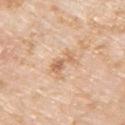<case>
<biopsy_status>not biopsied; imaged during a skin examination</biopsy_status>
<lesion_size>
  <long_diameter_mm_approx>3.0</long_diameter_mm_approx>
</lesion_size>
<automated_metrics>
  <area_mm2_approx>4.5</area_mm2_approx>
  <eccentricity>0.8</eccentricity>
  <shape_asymmetry>0.45</shape_asymmetry>
  <vs_skin_contrast_norm>6.5</vs_skin_contrast_norm>
  <nevus_likeness_0_100>0</nevus_likeness_0_100>
  <lesion_detection_confidence_0_100>100</lesion_detection_confidence_0_100>
</automated_metrics>
<patient>
  <sex>male</sex>
  <age_approx>75</age_approx>
</patient>
<image>
  <source>total-body photography crop</source>
  <field_of_view_mm>15</field_of_view_mm>
</image>
<site>arm</site>
<lighting>white-light</lighting>
</case>